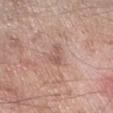Notes:
- follow-up · no biopsy performed (imaged during a skin exam)
- illumination · white-light illumination
- image · 15 mm crop, total-body photography
- location · the arm
- subject · male, roughly 60 years of age
- diameter · about 3 mm